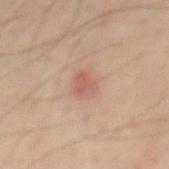Impression: Captured during whole-body skin photography for melanoma surveillance; the lesion was not biopsied. Clinical summary: Measured at roughly 2.5 mm in maximum diameter. From the mid back. A male subject, in their 60s. A close-up tile cropped from a whole-body skin photograph, about 15 mm across. The tile uses cross-polarized illumination.